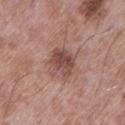image: total-body-photography crop, ~15 mm field of view
diameter: about 3.5 mm
tile lighting: white-light illumination
image-analysis metrics: a lesion area of about 8.5 mm², an outline eccentricity of about 0.65 (0 = round, 1 = elongated), and a symmetry-axis asymmetry near 0.2; a lesion color around L≈47 a*≈21 b*≈23 in CIELAB, about 12 CIELAB-L* units darker than the surrounding skin, and a normalized border contrast of about 8.5; a border-irregularity rating of about 2.5/10, internal color variation of about 4.5 on a 0–10 scale, and radial color variation of about 1.5; a nevus-likeness score of about 25/100 and lesion-presence confidence of about 100/100
site: the left lower leg
subject: male, aged 53–57